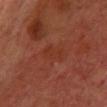The lesion was tiled from a total-body skin photograph and was not biopsied. Captured under cross-polarized illumination. Automated image analysis of the tile measured a lesion area of about 5 mm² and two-axis asymmetry of about 0.3. It also reported an average lesion color of about L≈30 a*≈25 b*≈28 (CIELAB) and a lesion-to-skin contrast of about 4.5 (normalized; higher = more distinct). The software also gave a border-irregularity index near 3.5/10 and a color-variation rating of about 1.5/10. A male subject, roughly 60 years of age. From the upper back. This image is a 15 mm lesion crop taken from a total-body photograph. About 3 mm across.